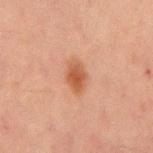This lesion was catalogued during total-body skin photography and was not selected for biopsy.
Automated image analysis of the tile measured a lesion area of about 6.5 mm², an outline eccentricity of about 0.8 (0 = round, 1 = elongated), and two-axis asymmetry of about 0.2. And it measured a mean CIELAB color near L≈45 a*≈21 b*≈30, a lesion–skin lightness drop of about 9, and a normalized lesion–skin contrast near 7.5. The software also gave border irregularity of about 2 on a 0–10 scale, a color-variation rating of about 3/10, and peripheral color asymmetry of about 1. The software also gave a classifier nevus-likeness of about 90/100 and a lesion-detection confidence of about 100/100.
This image is a 15 mm lesion crop taken from a total-body photograph.
The recorded lesion diameter is about 3.5 mm.
Imaged with cross-polarized lighting.
From the abdomen.
A male patient aged around 65.